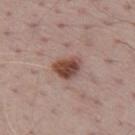| key | value |
|---|---|
| workup | total-body-photography surveillance lesion; no biopsy |
| location | the mid back |
| subject | male, aged 68 to 72 |
| imaging modality | ~15 mm crop, total-body skin-cancer survey |